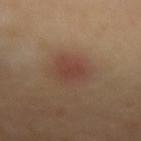follow-up=catalogued during a skin exam; not biopsied
patient=male, aged 53 to 57
anatomic site=the left forearm
automated metrics=an average lesion color of about L≈42 a*≈21 b*≈26 (CIELAB), roughly 7 lightness units darker than nearby skin, and a normalized border contrast of about 6; border irregularity of about 2 on a 0–10 scale and peripheral color asymmetry of about 0.5; an automated nevus-likeness rating near 10 out of 100
image=total-body-photography crop, ~15 mm field of view
lesion diameter=about 3.5 mm
tile lighting=cross-polarized illumination